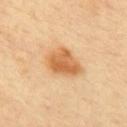A female subject, approximately 40 years of age. The lesion is located on the upper back. The lesion-visualizer software estimated a lesion area of about 12 mm² and a symmetry-axis asymmetry near 0.25. And it measured an average lesion color of about L≈65 a*≈23 b*≈43 (CIELAB) and a lesion–skin lightness drop of about 12. The analysis additionally found border irregularity of about 2 on a 0–10 scale, internal color variation of about 4.5 on a 0–10 scale, and radial color variation of about 1.5. The analysis additionally found a nevus-likeness score of about 100/100. Cropped from a total-body skin-imaging series; the visible field is about 15 mm.